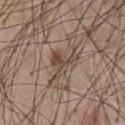– follow-up · imaged on a skin check; not biopsied
– location · the chest
– lesion size · about 4.5 mm
– imaging modality · ~15 mm tile from a whole-body skin photo
– patient · male, approximately 60 years of age